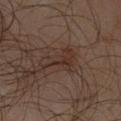The lesion was photographed on a routine skin check and not biopsied; there is no pathology result. A 15 mm close-up extracted from a 3D total-body photography capture. A male patient, aged approximately 65. From the right forearm. Longest diameter approximately 5 mm. Imaged with cross-polarized lighting. The total-body-photography lesion software estimated a shape eccentricity near 0.9. It also reported a border-irregularity index near 7.5/10 and a peripheral color-asymmetry measure near 1. The software also gave a classifier nevus-likeness of about 10/100 and lesion-presence confidence of about 95/100.